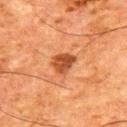– biopsy status · total-body-photography surveillance lesion; no biopsy
– automated lesion analysis · a lesion color around L≈40 a*≈26 b*≈35 in CIELAB, roughly 12 lightness units darker than nearby skin, and a normalized border contrast of about 9.5; internal color variation of about 3.5 on a 0–10 scale and peripheral color asymmetry of about 1.5
– diameter · about 3 mm
– acquisition · total-body-photography crop, ~15 mm field of view
– illumination · cross-polarized
– patient · male, aged around 65
– body site · the upper back